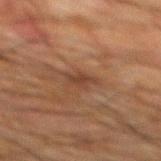Captured during whole-body skin photography for melanoma surveillance; the lesion was not biopsied.
On the mid back.
Imaged with cross-polarized lighting.
Cropped from a total-body skin-imaging series; the visible field is about 15 mm.
A male patient, roughly 65 years of age.